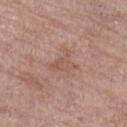workup — no biopsy performed (imaged during a skin exam)
image — ~15 mm tile from a whole-body skin photo
size — ≈3.5 mm
location — the leg
subject — female, in their mid- to late 80s or older
lighting — white-light illumination